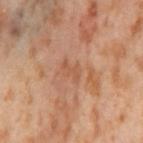- notes · imaged on a skin check; not biopsied
- image source · ~15 mm crop, total-body skin-cancer survey
- automated metrics · a lesion area of about 3 mm², an eccentricity of roughly 0.8, and two-axis asymmetry of about 0.5; border irregularity of about 5 on a 0–10 scale and radial color variation of about 0
- site · the left thigh
- patient · female, in their mid-50s